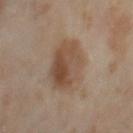Clinical impression: No biopsy was performed on this lesion — it was imaged during a full skin examination and was not determined to be concerning. Background: The tile uses cross-polarized illumination. Longest diameter approximately 5.5 mm. A female subject aged 48 to 52. From the leg. A region of skin cropped from a whole-body photographic capture, roughly 15 mm wide.